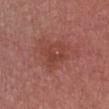Impression: No biopsy was performed on this lesion — it was imaged during a full skin examination and was not determined to be concerning. Clinical summary: Automated image analysis of the tile measured an area of roughly 8.5 mm², an eccentricity of roughly 0.6, and a symmetry-axis asymmetry near 0.2. The software also gave a lesion color around L≈43 a*≈27 b*≈27 in CIELAB, a lesion–skin lightness drop of about 6, and a normalized border contrast of about 5.5. A lesion tile, about 15 mm wide, cut from a 3D total-body photograph. On the front of the torso. The patient is a female aged 38–42.